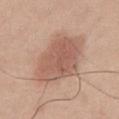No biopsy was performed on this lesion — it was imaged during a full skin examination and was not determined to be concerning. A 15 mm crop from a total-body photograph taken for skin-cancer surveillance. The lesion-visualizer software estimated a lesion color around L≈57 a*≈21 b*≈28 in CIELAB and a normalized lesion–skin contrast near 7. The analysis additionally found border irregularity of about 2.5 on a 0–10 scale and a within-lesion color-variation index near 3/10. The subject is a male aged around 30. Captured under white-light illumination. The recorded lesion diameter is about 8 mm. The lesion is on the chest.